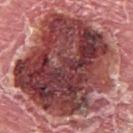Part of a total-body skin-imaging series; this lesion was reviewed on a skin check and was not flagged for biopsy. This image is a 15 mm lesion crop taken from a total-body photograph. A male patient aged 63 to 67. Captured under white-light illumination. Longest diameter approximately 12 mm. The lesion is located on the upper back. An algorithmic analysis of the crop reported a shape eccentricity near 0.45. The software also gave border irregularity of about 3.5 on a 0–10 scale, a color-variation rating of about 10/10, and radial color variation of about 4. The software also gave a nevus-likeness score of about 0/100 and a detector confidence of about 100 out of 100 that the crop contains a lesion.